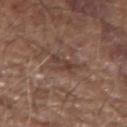Recorded during total-body skin imaging; not selected for excision or biopsy. From the left forearm. The tile uses white-light illumination. A male patient approximately 75 years of age. This image is a 15 mm lesion crop taken from a total-body photograph.